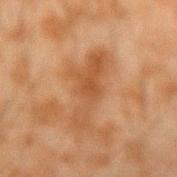Assessment:
The lesion was photographed on a routine skin check and not biopsied; there is no pathology result.
Image and clinical context:
The lesion is located on the right forearm. A 15 mm close-up extracted from a 3D total-body photography capture. About 7.5 mm across. A male subject, aged around 45.